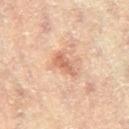notes=imaged on a skin check; not biopsied | diameter=about 3.5 mm | lighting=cross-polarized | site=the left leg | image=total-body-photography crop, ~15 mm field of view | patient=female, approximately 80 years of age.